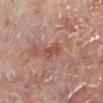biopsy status = imaged on a skin check; not biopsied | tile lighting = cross-polarized | size = ~3 mm (longest diameter) | anatomic site = the left lower leg | subject = male, aged 58–62 | image = ~15 mm crop, total-body skin-cancer survey.